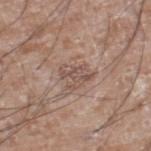notes: no biopsy performed (imaged during a skin exam)
illumination: white-light illumination
site: the right lower leg
imaging modality: ~15 mm crop, total-body skin-cancer survey
subject: male, in their 60s
lesion diameter: ≈3.5 mm